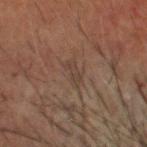Recorded during total-body skin imaging; not selected for excision or biopsy.
The recorded lesion diameter is about 3 mm.
Cropped from a whole-body photographic skin survey; the tile spans about 15 mm.
The lesion is located on the head or neck.
This is a cross-polarized tile.
The patient is a male in their 70s.